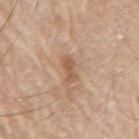Imaged during a routine full-body skin examination; the lesion was not biopsied and no histopathology is available.
Automated tile analysis of the lesion measured border irregularity of about 3 on a 0–10 scale, a within-lesion color-variation index near 1/10, and a peripheral color-asymmetry measure near 0. It also reported an automated nevus-likeness rating near 0 out of 100 and lesion-presence confidence of about 100/100.
Located on the arm.
The recorded lesion diameter is about 3.5 mm.
A male patient about 65 years old.
Captured under white-light illumination.
Cropped from a total-body skin-imaging series; the visible field is about 15 mm.